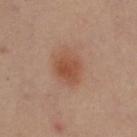Located on the right upper arm. A female patient, roughly 40 years of age. A 15 mm crop from a total-body photograph taken for skin-cancer surveillance.